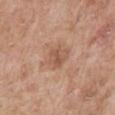Notes:
- biopsy status · no biopsy performed (imaged during a skin exam)
- body site · the chest
- subject · male, aged 58 to 62
- acquisition · ~15 mm crop, total-body skin-cancer survey
- tile lighting · white-light
- lesion diameter · ≈2.5 mm